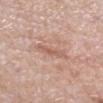Background: Captured under white-light illumination. The total-body-photography lesion software estimated an area of roughly 4 mm² and an eccentricity of roughly 0.95. And it measured a within-lesion color-variation index near 1.5/10 and a peripheral color-asymmetry measure near 0.5. The analysis additionally found a classifier nevus-likeness of about 0/100 and a lesion-detection confidence of about 90/100. A close-up tile cropped from a whole-body skin photograph, about 15 mm across. From the left lower leg. A female subject, aged 68 to 72. Longest diameter approximately 3.5 mm.